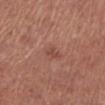Imaged during a routine full-body skin examination; the lesion was not biopsied and no histopathology is available. From the left lower leg. A female patient in their mid-60s. A roughly 15 mm field-of-view crop from a total-body skin photograph.